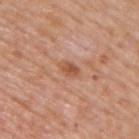Clinical impression: Imaged during a routine full-body skin examination; the lesion was not biopsied and no histopathology is available. Context: Cropped from a whole-body photographic skin survey; the tile spans about 15 mm. Captured under white-light illumination. Longest diameter approximately 3 mm. On the back. A male patient in their mid- to late 70s.